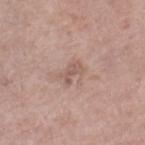{
  "biopsy_status": "not biopsied; imaged during a skin examination",
  "automated_metrics": {
    "area_mm2_approx": 3.5,
    "eccentricity": 0.85,
    "shape_asymmetry": 0.3,
    "nevus_likeness_0_100": 0,
    "lesion_detection_confidence_0_100": 100
  },
  "lighting": "white-light",
  "image": {
    "source": "total-body photography crop",
    "field_of_view_mm": 15
  },
  "site": "right lower leg",
  "patient": {
    "sex": "female",
    "age_approx": 65
  }
}